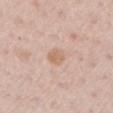  biopsy_status: not biopsied; imaged during a skin examination
  lighting: white-light
  lesion_size:
    long_diameter_mm_approx: 2.5
  site: left upper arm
  patient:
    sex: male
    age_approx: 60
  image:
    source: total-body photography crop
    field_of_view_mm: 15